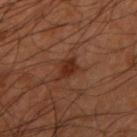Image and clinical context:
The lesion is located on the right upper arm. A close-up tile cropped from a whole-body skin photograph, about 15 mm across. This is a cross-polarized tile. The patient is a male in their mid- to late 40s. Measured at roughly 3 mm in maximum diameter.